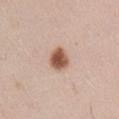Q: Is there a histopathology result?
A: total-body-photography surveillance lesion; no biopsy
Q: What are the patient's age and sex?
A: male, aged approximately 30
Q: What did automated image analysis measure?
A: an area of roughly 6 mm², an outline eccentricity of about 0.55 (0 = round, 1 = elongated), and a symmetry-axis asymmetry near 0.15; a classifier nevus-likeness of about 100/100 and a detector confidence of about 100 out of 100 that the crop contains a lesion
Q: What kind of image is this?
A: 15 mm crop, total-body photography
Q: What is the anatomic site?
A: the right upper arm
Q: How large is the lesion?
A: ≈3 mm